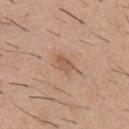| key | value |
|---|---|
| biopsy status | catalogued during a skin exam; not biopsied |
| body site | the chest |
| image | total-body-photography crop, ~15 mm field of view |
| subject | male, aged 38–42 |
| lighting | white-light illumination |